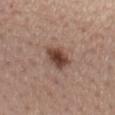Notes:
• biopsy status — imaged on a skin check; not biopsied
• site — the mid back
• patient — female, aged around 65
• imaging modality — ~15 mm crop, total-body skin-cancer survey